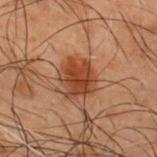<tbp_lesion>
<biopsy_status>not biopsied; imaged during a skin examination</biopsy_status>
<lighting>cross-polarized</lighting>
<site>upper back</site>
<patient>
  <sex>male</sex>
  <age_approx>50</age_approx>
</patient>
<lesion_size>
  <long_diameter_mm_approx>4.0</long_diameter_mm_approx>
</lesion_size>
<image>
  <source>total-body photography crop</source>
  <field_of_view_mm>15</field_of_view_mm>
</image>
</tbp_lesion>